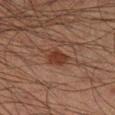workup: no biopsy performed (imaged during a skin exam) | body site: the right lower leg | automated lesion analysis: a footprint of about 5 mm² and an outline eccentricity of about 0.65 (0 = round, 1 = elongated) | size: ~3 mm (longest diameter) | image: ~15 mm tile from a whole-body skin photo | patient: male, roughly 50 years of age.